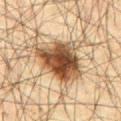This lesion was catalogued during total-body skin photography and was not selected for biopsy.
Cropped from a whole-body photographic skin survey; the tile spans about 15 mm.
The tile uses cross-polarized illumination.
From the abdomen.
The patient is a male approximately 65 years of age.